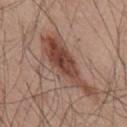The lesion was tiled from a total-body skin photograph and was not biopsied. A 15 mm close-up extracted from a 3D total-body photography capture. Located on the upper back. Longest diameter approximately 10 mm. Automated image analysis of the tile measured internal color variation of about 7.5 on a 0–10 scale and peripheral color asymmetry of about 3. It also reported a nevus-likeness score of about 35/100 and a lesion-detection confidence of about 95/100. The patient is a male approximately 55 years of age.